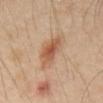Impression: Part of a total-body skin-imaging series; this lesion was reviewed on a skin check and was not flagged for biopsy. Background: A male patient in their mid-40s. The lesion's longest dimension is about 5.5 mm. Captured under cross-polarized illumination. An algorithmic analysis of the crop reported an outline eccentricity of about 0.85 (0 = round, 1 = elongated). And it measured a mean CIELAB color near L≈53 a*≈19 b*≈31, roughly 9 lightness units darker than nearby skin, and a normalized border contrast of about 7. The analysis additionally found a within-lesion color-variation index near 5/10 and radial color variation of about 1.5. Located on the abdomen. A 15 mm crop from a total-body photograph taken for skin-cancer surveillance.